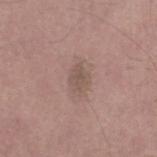Imaged during a routine full-body skin examination; the lesion was not biopsied and no histopathology is available. Automated tile analysis of the lesion measured a border-irregularity index near 3/10 and radial color variation of about 0.5. The tile uses white-light illumination. A male subject, roughly 45 years of age. A 15 mm crop from a total-body photograph taken for skin-cancer surveillance. Located on the left lower leg. Longest diameter approximately 3 mm.